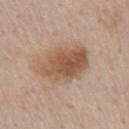The lesion was photographed on a routine skin check and not biopsied; there is no pathology result. A region of skin cropped from a whole-body photographic capture, roughly 15 mm wide. Located on the mid back. A male patient, aged 58–62.